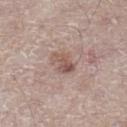follow-up = no biopsy performed (imaged during a skin exam) | automated lesion analysis = a footprint of about 6.5 mm² and a symmetry-axis asymmetry near 0.25; an average lesion color of about L≈55 a*≈17 b*≈23 (CIELAB), about 9 CIELAB-L* units darker than the surrounding skin, and a normalized border contrast of about 6.5 | patient = male, in their mid-60s | illumination = white-light illumination | site = the left lower leg | lesion size = ≈3.5 mm | image source = 15 mm crop, total-body photography.